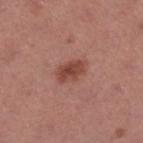Q: Is there a histopathology result?
A: no biopsy performed (imaged during a skin exam)
Q: Who is the patient?
A: female, about 55 years old
Q: What did automated image analysis measure?
A: about 10 CIELAB-L* units darker than the surrounding skin and a lesion-to-skin contrast of about 8 (normalized; higher = more distinct)
Q: What is the anatomic site?
A: the right lower leg
Q: How was this image acquired?
A: total-body-photography crop, ~15 mm field of view